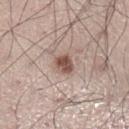{"patient": {"sex": "male", "age_approx": 30}, "automated_metrics": {"area_mm2_approx": 4.0, "eccentricity": 0.65, "shape_asymmetry": 0.2, "vs_skin_darker_L": 15.0, "vs_skin_contrast_norm": 10.0, "border_irregularity_0_10": 1.5, "peripheral_color_asymmetry": 1.0, "nevus_likeness_0_100": 90, "lesion_detection_confidence_0_100": 100}, "site": "left lower leg", "image": {"source": "total-body photography crop", "field_of_view_mm": 15}, "lighting": "white-light"}